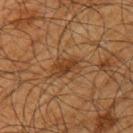* site — the left upper arm
* image source — total-body-photography crop, ~15 mm field of view
* subject — male, about 65 years old